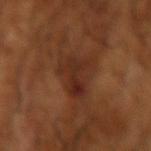Notes:
- notes — catalogued during a skin exam; not biopsied
- site — the right forearm
- lesion diameter — about 4.5 mm
- subject — male, approximately 65 years of age
- lighting — cross-polarized illumination
- imaging modality — ~15 mm tile from a whole-body skin photo
- TBP lesion metrics — an area of roughly 11 mm² and a shape eccentricity near 0.45; a lesion color around L≈29 a*≈23 b*≈28 in CIELAB, roughly 7 lightness units darker than nearby skin, and a lesion-to-skin contrast of about 7 (normalized; higher = more distinct); a border-irregularity rating of about 5.5/10 and radial color variation of about 1.5; a nevus-likeness score of about 0/100